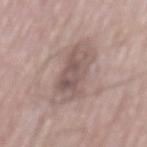  biopsy_status: not biopsied; imaged during a skin examination
  image:
    source: total-body photography crop
    field_of_view_mm: 15
  automated_metrics:
    eccentricity: 0.9
    shape_asymmetry: 0.2
    cielab_L: 55
    cielab_a: 15
    cielab_b: 20
    vs_skin_darker_L: 10.0
    border_irregularity_0_10: 3.0
    color_variation_0_10: 4.5
    peripheral_color_asymmetry: 1.5
  lighting: white-light
  patient:
    sex: male
    age_approx: 65
  site: mid back
  lesion_size:
    long_diameter_mm_approx: 7.0